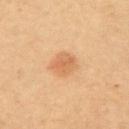Impression:
Part of a total-body skin-imaging series; this lesion was reviewed on a skin check and was not flagged for biopsy.
Context:
A female patient aged approximately 40. From the left upper arm. A lesion tile, about 15 mm wide, cut from a 3D total-body photograph. Longest diameter approximately 2.5 mm. The tile uses cross-polarized illumination.